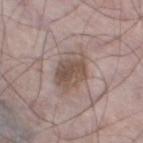| key | value |
|---|---|
| acquisition | ~15 mm tile from a whole-body skin photo |
| location | the left thigh |
| patient | male, aged around 65 |